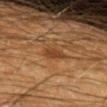Part of a total-body skin-imaging series; this lesion was reviewed on a skin check and was not flagged for biopsy.
The subject is a male approximately 60 years of age.
Located on the arm.
This image is a 15 mm lesion crop taken from a total-body photograph.